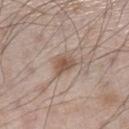Part of a total-body skin-imaging series; this lesion was reviewed on a skin check and was not flagged for biopsy. The lesion is on the left lower leg. A lesion tile, about 15 mm wide, cut from a 3D total-body photograph. The subject is a male about 60 years old.